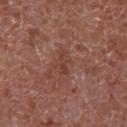Case summary:
• image — 15 mm crop, total-body photography
• illumination — white-light illumination
• image-analysis metrics — an area of roughly 3.5 mm², a shape eccentricity near 0.9, and a shape-asymmetry score of about 0.45 (0 = symmetric); radial color variation of about 0; a detector confidence of about 100 out of 100 that the crop contains a lesion
• location — the abdomen
• patient — male, aged 63–67
• lesion size — about 3 mm